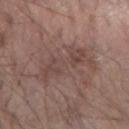Acquisition and patient details: Cropped from a total-body skin-imaging series; the visible field is about 15 mm. This is a white-light tile. On the right forearm. A male patient roughly 70 years of age. Longest diameter approximately 8.5 mm.